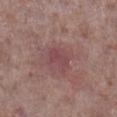The lesion was photographed on a routine skin check and not biopsied; there is no pathology result. This image is a 15 mm lesion crop taken from a total-body photograph. Located on the right lower leg. Captured under white-light illumination. About 2.5 mm across. The patient is a male approximately 70 years of age.